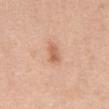Clinical impression: Imaged during a routine full-body skin examination; the lesion was not biopsied and no histopathology is available. Acquisition and patient details: The patient is a female in their 40s. The recorded lesion diameter is about 2.5 mm. On the abdomen. The total-body-photography lesion software estimated a footprint of about 4 mm² and a shape eccentricity near 0.8. The analysis additionally found an average lesion color of about L≈62 a*≈23 b*≈33 (CIELAB) and roughly 10 lightness units darker than nearby skin. It also reported a border-irregularity index near 3/10, a within-lesion color-variation index near 3/10, and peripheral color asymmetry of about 1. A roughly 15 mm field-of-view crop from a total-body skin photograph. The tile uses white-light illumination.